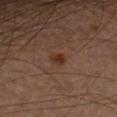Q: Was a biopsy performed?
A: no biopsy performed (imaged during a skin exam)
Q: Where on the body is the lesion?
A: the arm
Q: How was this image acquired?
A: total-body-photography crop, ~15 mm field of view
Q: What are the patient's age and sex?
A: male, aged approximately 65
Q: Lesion size?
A: ~2 mm (longest diameter)